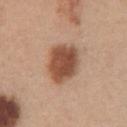biopsy_status: not biopsied; imaged during a skin examination